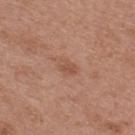Q: Is there a histopathology result?
A: catalogued during a skin exam; not biopsied
Q: What is the lesion's diameter?
A: ≈2.5 mm
Q: Where on the body is the lesion?
A: the upper back
Q: Who is the patient?
A: female, aged 38–42
Q: Automated lesion metrics?
A: an automated nevus-likeness rating near 10 out of 100 and a detector confidence of about 100 out of 100 that the crop contains a lesion
Q: Illumination type?
A: white-light
Q: What kind of image is this?
A: ~15 mm crop, total-body skin-cancer survey